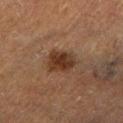<tbp_lesion>
  <biopsy_status>not biopsied; imaged during a skin examination</biopsy_status>
  <site>leg</site>
  <image>
    <source>total-body photography crop</source>
    <field_of_view_mm>15</field_of_view_mm>
  </image>
  <lesion_size>
    <long_diameter_mm_approx>3.5</long_diameter_mm_approx>
  </lesion_size>
  <patient>
    <sex>male</sex>
    <age_approx>75</age_approx>
  </patient>
</tbp_lesion>